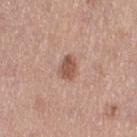The lesion was tiled from a total-body skin photograph and was not biopsied.
The lesion is located on the left thigh.
A female subject, about 40 years old.
A close-up tile cropped from a whole-body skin photograph, about 15 mm across.
Imaged with white-light lighting.
Automated image analysis of the tile measured a lesion area of about 5 mm², an eccentricity of roughly 0.8, and two-axis asymmetry of about 0.25. And it measured an average lesion color of about L≈54 a*≈22 b*≈28 (CIELAB), roughly 12 lightness units darker than nearby skin, and a normalized border contrast of about 8. And it measured a border-irregularity rating of about 2.5/10, a color-variation rating of about 2.5/10, and radial color variation of about 1. The software also gave a classifier nevus-likeness of about 85/100 and a lesion-detection confidence of about 100/100.
Approximately 3 mm at its widest.